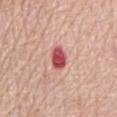Q: Is there a histopathology result?
A: total-body-photography surveillance lesion; no biopsy
Q: Lesion location?
A: the chest
Q: Lesion size?
A: ~3 mm (longest diameter)
Q: Patient demographics?
A: male, aged approximately 80
Q: What kind of image is this?
A: total-body-photography crop, ~15 mm field of view